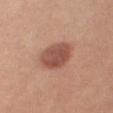Case summary:
• workup · no biopsy performed (imaged during a skin exam)
• anatomic site · the abdomen
• subject · female, in their 40s
• lighting · cross-polarized illumination
• lesion size · ≈5 mm
• imaging modality · 15 mm crop, total-body photography